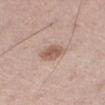Q: Is there a histopathology result?
A: imaged on a skin check; not biopsied
Q: What is the anatomic site?
A: the abdomen
Q: What did automated image analysis measure?
A: a footprint of about 6.5 mm² and two-axis asymmetry of about 0.2; about 11 CIELAB-L* units darker than the surrounding skin and a normalized border contrast of about 7.5; a classifier nevus-likeness of about 80/100 and a detector confidence of about 100 out of 100 that the crop contains a lesion
Q: What is the imaging modality?
A: ~15 mm tile from a whole-body skin photo
Q: Who is the patient?
A: male, in their 70s